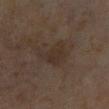Assessment: The lesion was photographed on a routine skin check and not biopsied; there is no pathology result. Image and clinical context: Located on the right lower leg. This image is a 15 mm lesion crop taken from a total-body photograph. Automated tile analysis of the lesion measured a lesion area of about 7.5 mm², an eccentricity of roughly 0.25, and two-axis asymmetry of about 0.45. The software also gave a border-irregularity rating of about 5/10, a within-lesion color-variation index near 1.5/10, and radial color variation of about 0.5. And it measured a classifier nevus-likeness of about 0/100 and lesion-presence confidence of about 100/100. Captured under cross-polarized illumination. The patient is a female about 60 years old.